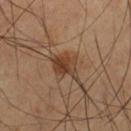Assessment:
The lesion was tiled from a total-body skin photograph and was not biopsied.
Acquisition and patient details:
Captured under cross-polarized illumination. From the right thigh. The lesion's longest dimension is about 4 mm. A close-up tile cropped from a whole-body skin photograph, about 15 mm across. A male patient in their 60s.